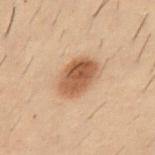| key | value |
|---|---|
| follow-up | no biopsy performed (imaged during a skin exam) |
| automated lesion analysis | an area of roughly 12 mm², an outline eccentricity of about 0.7 (0 = round, 1 = elongated), and a symmetry-axis asymmetry near 0.15; a lesion color around L≈45 a*≈18 b*≈29 in CIELAB, roughly 12 lightness units darker than nearby skin, and a lesion-to-skin contrast of about 9.5 (normalized; higher = more distinct); a border-irregularity rating of about 1.5/10, a within-lesion color-variation index near 4.5/10, and radial color variation of about 1.5; a lesion-detection confidence of about 100/100 |
| patient | male, about 30 years old |
| lesion diameter | about 4.5 mm |
| imaging modality | ~15 mm crop, total-body skin-cancer survey |
| lighting | cross-polarized |
| body site | the front of the torso |